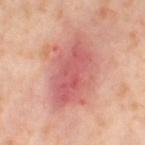Assessment:
Captured during whole-body skin photography for melanoma surveillance; the lesion was not biopsied.
Clinical summary:
The lesion is located on the left thigh. A female patient about 55 years old. A roughly 15 mm field-of-view crop from a total-body skin photograph.